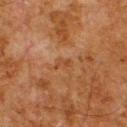biopsy_status: not biopsied; imaged during a skin examination
lesion_size:
  long_diameter_mm_approx: 2.5
lighting: cross-polarized
site: upper back
image:
  source: total-body photography crop
  field_of_view_mm: 15
patient:
  sex: male
  age_approx: 80